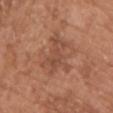This lesion was catalogued during total-body skin photography and was not selected for biopsy.
Cropped from a whole-body photographic skin survey; the tile spans about 15 mm.
A male subject aged approximately 65.
Automated image analysis of the tile measured a mean CIELAB color near L≈48 a*≈23 b*≈31, a lesion–skin lightness drop of about 7, and a normalized lesion–skin contrast near 5.5. The analysis additionally found border irregularity of about 9 on a 0–10 scale, a within-lesion color-variation index near 2/10, and a peripheral color-asymmetry measure near 0.5.
On the chest.
Captured under white-light illumination.